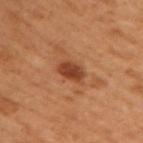• follow-up · catalogued during a skin exam; not biopsied
• image source · total-body-photography crop, ~15 mm field of view
• body site · the back
• illumination · cross-polarized
• automated lesion analysis · an outline eccentricity of about 0.8 (0 = round, 1 = elongated) and a symmetry-axis asymmetry near 0.15; a border-irregularity index near 1.5/10, a color-variation rating of about 2.5/10, and a peripheral color-asymmetry measure near 1
• patient · male, aged 48–52